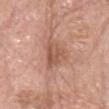| feature | finding |
|---|---|
| workup | imaged on a skin check; not biopsied |
| lesion diameter | about 4 mm |
| TBP lesion metrics | a mean CIELAB color near L≈55 a*≈23 b*≈29, about 9 CIELAB-L* units darker than the surrounding skin, and a normalized lesion–skin contrast near 6.5; a nevus-likeness score of about 0/100 and a lesion-detection confidence of about 100/100 |
| tile lighting | white-light illumination |
| subject | male, about 65 years old |
| imaging modality | total-body-photography crop, ~15 mm field of view |
| location | the head or neck |